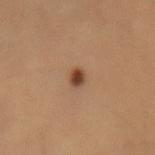Impression: Captured during whole-body skin photography for melanoma surveillance; the lesion was not biopsied. Background: The subject is a male aged around 35. A close-up tile cropped from a whole-body skin photograph, about 15 mm across. Captured under cross-polarized illumination. The recorded lesion diameter is about 2 mm. The total-body-photography lesion software estimated a lesion color around L≈40 a*≈21 b*≈30 in CIELAB, a lesion–skin lightness drop of about 14, and a normalized border contrast of about 11. The software also gave a border-irregularity index near 2.5/10, a within-lesion color-variation index near 1.5/10, and a peripheral color-asymmetry measure near 0.5. The software also gave a classifier nevus-likeness of about 100/100 and lesion-presence confidence of about 100/100. The lesion is on the mid back.